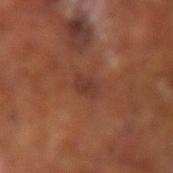Impression: Imaged during a routine full-body skin examination; the lesion was not biopsied and no histopathology is available. Clinical summary: The subject is a male aged 63 to 67. The lesion is located on the left arm. Measured at roughly 2.5 mm in maximum diameter. An algorithmic analysis of the crop reported an area of roughly 3.5 mm² and an outline eccentricity of about 0.8 (0 = round, 1 = elongated). The analysis additionally found an average lesion color of about L≈35 a*≈23 b*≈26 (CIELAB) and about 7 CIELAB-L* units darker than the surrounding skin. It also reported a border-irregularity rating of about 2.5/10 and radial color variation of about 0.5. The software also gave an automated nevus-likeness rating near 5 out of 100 and lesion-presence confidence of about 100/100. A close-up tile cropped from a whole-body skin photograph, about 15 mm across. Imaged with cross-polarized lighting.